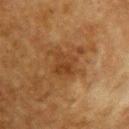Case summary:
- biopsy status — imaged on a skin check; not biopsied
- imaging modality — 15 mm crop, total-body photography
- location — the upper back
- patient — female, aged 53–57